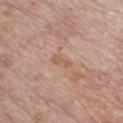This lesion was catalogued during total-body skin photography and was not selected for biopsy.
The subject is a female aged 68 to 72.
A close-up tile cropped from a whole-body skin photograph, about 15 mm across.
The lesion is on the chest.